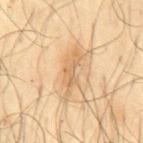The subject is a male aged 63 to 67.
Located on the mid back.
A roughly 15 mm field-of-view crop from a total-body skin photograph.